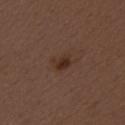<lesion>
  <biopsy_status>not biopsied; imaged during a skin examination</biopsy_status>
</lesion>